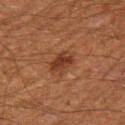Part of a total-body skin-imaging series; this lesion was reviewed on a skin check and was not flagged for biopsy. This is a cross-polarized tile. Located on the right thigh. A region of skin cropped from a whole-body photographic capture, roughly 15 mm wide. A male subject in their 60s. Measured at roughly 3 mm in maximum diameter.